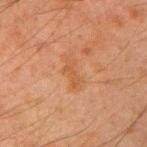Impression: Part of a total-body skin-imaging series; this lesion was reviewed on a skin check and was not flagged for biopsy. Background: The subject is a male approximately 35 years of age. A close-up tile cropped from a whole-body skin photograph, about 15 mm across. The lesion is on the right upper arm. The lesion's longest dimension is about 4 mm.